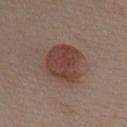Case summary:
• workup — total-body-photography surveillance lesion; no biopsy
• size — ~4.5 mm (longest diameter)
• patient — female, approximately 35 years of age
• automated metrics — two-axis asymmetry of about 0.2; a border-irregularity rating of about 2/10, a within-lesion color-variation index near 3/10, and peripheral color asymmetry of about 1
• anatomic site — the chest
• tile lighting — white-light
• imaging modality — ~15 mm crop, total-body skin-cancer survey